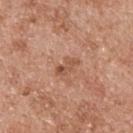  biopsy_status: not biopsied; imaged during a skin examination
  automated_metrics:
    nevus_likeness_0_100: 5
    lesion_detection_confidence_0_100: 100
  image:
    source: total-body photography crop
    field_of_view_mm: 15
  site: back
  lesion_size:
    long_diameter_mm_approx: 2.5
  patient:
    sex: male
    age_approx: 55
  lighting: white-light